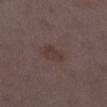The lesion was tiled from a total-body skin photograph and was not biopsied. A female patient roughly 30 years of age. This image is a 15 mm lesion crop taken from a total-body photograph. Captured under white-light illumination. The lesion is on the left lower leg. The total-body-photography lesion software estimated an area of roughly 5 mm² and an eccentricity of roughly 0.65. And it measured a mean CIELAB color near L≈36 a*≈14 b*≈19 and about 5 CIELAB-L* units darker than the surrounding skin. And it measured radial color variation of about 0.5. The software also gave a classifier nevus-likeness of about 0/100 and a lesion-detection confidence of about 100/100. Longest diameter approximately 2.5 mm.